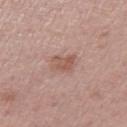workup — total-body-photography surveillance lesion; no biopsy | lesion size — about 3 mm | image source — total-body-photography crop, ~15 mm field of view | subject — male, roughly 55 years of age | anatomic site — the left thigh.